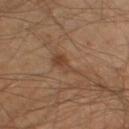Impression:
Imaged during a routine full-body skin examination; the lesion was not biopsied and no histopathology is available.
Clinical summary:
A male subject in their mid-40s. The recorded lesion diameter is about 4.5 mm. The total-body-photography lesion software estimated a mean CIELAB color near L≈42 a*≈18 b*≈30. The software also gave lesion-presence confidence of about 100/100. Located on the right thigh. A 15 mm close-up tile from a total-body photography series done for melanoma screening. Captured under cross-polarized illumination.